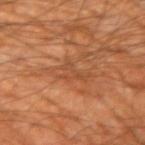No biopsy was performed on this lesion — it was imaged during a full skin examination and was not determined to be concerning.
Captured under cross-polarized illumination.
On the right upper arm.
The recorded lesion diameter is about 4.5 mm.
The subject is a male in their 60s.
A 15 mm crop from a total-body photograph taken for skin-cancer surveillance.